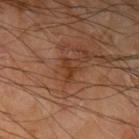Part of a total-body skin-imaging series; this lesion was reviewed on a skin check and was not flagged for biopsy. The lesion is located on the left upper arm. Captured under cross-polarized illumination. A roughly 15 mm field-of-view crop from a total-body skin photograph. A male subject in their mid-60s. Approximately 2.5 mm at its widest.